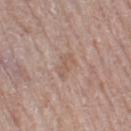Q: Is there a histopathology result?
A: no biopsy performed (imaged during a skin exam)
Q: What is the anatomic site?
A: the left thigh
Q: Illumination type?
A: white-light
Q: Who is the patient?
A: female, aged approximately 65
Q: What is the imaging modality?
A: 15 mm crop, total-body photography
Q: How large is the lesion?
A: about 3 mm
Q: What did automated image analysis measure?
A: a footprint of about 2.5 mm²; a mean CIELAB color near L≈56 a*≈17 b*≈26 and a lesion–skin lightness drop of about 6; internal color variation of about 0 on a 0–10 scale and radial color variation of about 0; a nevus-likeness score of about 0/100 and a lesion-detection confidence of about 100/100